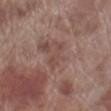This lesion was catalogued during total-body skin photography and was not selected for biopsy. Imaged with white-light lighting. Cropped from a whole-body photographic skin survey; the tile spans about 15 mm. A male subject about 70 years old. The lesion-visualizer software estimated a mean CIELAB color near L≈47 a*≈19 b*≈22. Approximately 4 mm at its widest. On the leg.